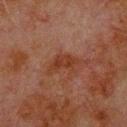This lesion was catalogued during total-body skin photography and was not selected for biopsy.
This is a cross-polarized tile.
The recorded lesion diameter is about 4 mm.
A male patient roughly 80 years of age.
Located on the upper back.
A lesion tile, about 15 mm wide, cut from a 3D total-body photograph.